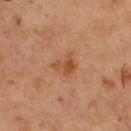Notes:
– notes: imaged on a skin check; not biopsied
– lighting: cross-polarized
– site: the upper back
– automated metrics: a lesion area of about 5.5 mm², an eccentricity of roughly 0.55, and two-axis asymmetry of about 0.4; a border-irregularity index near 4/10, a color-variation rating of about 4.5/10, and peripheral color asymmetry of about 1.5
– image source: 15 mm crop, total-body photography
– lesion diameter: ≈3 mm
– patient: male, aged around 55